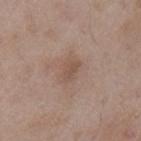<record>
<site>left upper arm</site>
<image>
  <source>total-body photography crop</source>
  <field_of_view_mm>15</field_of_view_mm>
</image>
<lesion_size>
  <long_diameter_mm_approx>2.5</long_diameter_mm_approx>
</lesion_size>
<patient>
  <sex>male</sex>
  <age_approx>50</age_approx>
</patient>
<lighting>white-light</lighting>
</record>